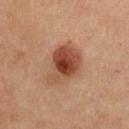Case summary:
– workup — total-body-photography surveillance lesion; no biopsy
– acquisition — 15 mm crop, total-body photography
– tile lighting — cross-polarized
– subject — female, about 45 years old
– site — the chest
– lesion size — ~5.5 mm (longest diameter)